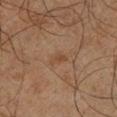• notes — total-body-photography surveillance lesion; no biopsy
• anatomic site — the left lower leg
• image source — 15 mm crop, total-body photography
• TBP lesion metrics — a mean CIELAB color near L≈36 a*≈16 b*≈26; a border-irregularity index near 3.5/10 and a within-lesion color-variation index near 0.5/10; a nevus-likeness score of about 0/100 and a detector confidence of about 100 out of 100 that the crop contains a lesion
• lesion size — ≈2.5 mm
• patient — male, in their mid-60s
• lighting — cross-polarized illumination